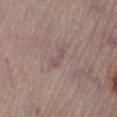Q: How was this image acquired?
A: ~15 mm crop, total-body skin-cancer survey
Q: Where on the body is the lesion?
A: the left lower leg
Q: How large is the lesion?
A: ~2.5 mm (longest diameter)
Q: What lighting was used for the tile?
A: white-light illumination
Q: What did automated image analysis measure?
A: an area of roughly 2.5 mm² and a shape-asymmetry score of about 0.55 (0 = symmetric); a within-lesion color-variation index near 0/10 and peripheral color asymmetry of about 0
Q: What are the patient's age and sex?
A: male, aged approximately 55